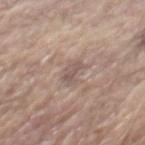{
  "biopsy_status": "not biopsied; imaged during a skin examination",
  "site": "mid back",
  "lesion_size": {
    "long_diameter_mm_approx": 3.0
  },
  "automated_metrics": {
    "cielab_L": 54,
    "cielab_a": 15,
    "cielab_b": 21,
    "vs_skin_darker_L": 8.0,
    "vs_skin_contrast_norm": 6.0
  },
  "image": {
    "source": "total-body photography crop",
    "field_of_view_mm": 15
  },
  "patient": {
    "sex": "male",
    "age_approx": 65
  },
  "lighting": "white-light"
}